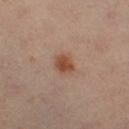Assessment: No biopsy was performed on this lesion — it was imaged during a full skin examination and was not determined to be concerning. Context: The total-body-photography lesion software estimated an automated nevus-likeness rating near 100 out of 100 and lesion-presence confidence of about 100/100. The lesion is on the left leg. The patient is a female roughly 40 years of age. Approximately 3 mm at its widest. A lesion tile, about 15 mm wide, cut from a 3D total-body photograph.